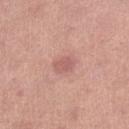Q: Patient demographics?
A: female, aged 58–62
Q: Lesion size?
A: ≈2.5 mm
Q: What kind of image is this?
A: total-body-photography crop, ~15 mm field of view
Q: Where on the body is the lesion?
A: the leg